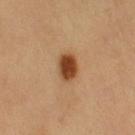The lesion was tiled from a total-body skin photograph and was not biopsied. A 15 mm close-up tile from a total-body photography series done for melanoma screening. A male patient, about 60 years old. From the left upper arm.